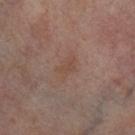Image and clinical context:
On the right thigh. An algorithmic analysis of the crop reported a lesion color around L≈47 a*≈18 b*≈25 in CIELAB and a lesion-to-skin contrast of about 4.5 (normalized; higher = more distinct). The analysis additionally found a border-irregularity index near 3/10, a color-variation rating of about 1/10, and radial color variation of about 0.5. The patient is a female approximately 55 years of age. Measured at roughly 2.5 mm in maximum diameter. Cropped from a total-body skin-imaging series; the visible field is about 15 mm.